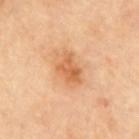This lesion was catalogued during total-body skin photography and was not selected for biopsy.
Imaged with cross-polarized lighting.
On the mid back.
A 15 mm close-up extracted from a 3D total-body photography capture.
Approximately 4 mm at its widest.